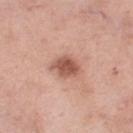<lesion>
<biopsy_status>not biopsied; imaged during a skin examination</biopsy_status>
<lesion_size>
  <long_diameter_mm_approx>3.5</long_diameter_mm_approx>
</lesion_size>
<site>left thigh</site>
<image>
  <source>total-body photography crop</source>
  <field_of_view_mm>15</field_of_view_mm>
</image>
<patient>
  <sex>female</sex>
  <age_approx>55</age_approx>
</patient>
</lesion>